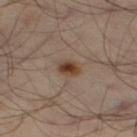This is a cross-polarized tile.
Longest diameter approximately 3 mm.
From the front of the torso.
A 15 mm close-up extracted from a 3D total-body photography capture.
A male subject, roughly 50 years of age.